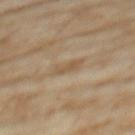The lesion was photographed on a routine skin check and not biopsied; there is no pathology result.
A female subject, aged around 75.
A close-up tile cropped from a whole-body skin photograph, about 15 mm across.
Captured under cross-polarized illumination.
On the chest.